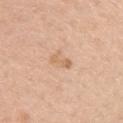No biopsy was performed on this lesion — it was imaged during a full skin examination and was not determined to be concerning. Captured under white-light illumination. The lesion is located on the left upper arm. A female patient aged approximately 40. Cropped from a whole-body photographic skin survey; the tile spans about 15 mm. Automated image analysis of the tile measured an outline eccentricity of about 0.95 (0 = round, 1 = elongated). And it measured an average lesion color of about L≈65 a*≈20 b*≈35 (CIELAB), about 8 CIELAB-L* units darker than the surrounding skin, and a lesion-to-skin contrast of about 6 (normalized; higher = more distinct).